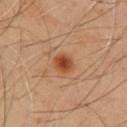notes — total-body-photography surveillance lesion; no biopsy
subject — male, in their mid- to late 50s
image source — total-body-photography crop, ~15 mm field of view
size — ~2.5 mm (longest diameter)
anatomic site — the chest